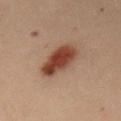{"biopsy_status": "not biopsied; imaged during a skin examination", "patient": {"sex": "female", "age_approx": 30}, "lighting": "cross-polarized", "site": "abdomen", "image": {"source": "total-body photography crop", "field_of_view_mm": 15}, "lesion_size": {"long_diameter_mm_approx": 5.0}}